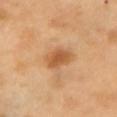<lesion>
  <biopsy_status>not biopsied; imaged during a skin examination</biopsy_status>
  <lesion_size>
    <long_diameter_mm_approx>3.5</long_diameter_mm_approx>
  </lesion_size>
  <image>
    <source>total-body photography crop</source>
    <field_of_view_mm>15</field_of_view_mm>
  </image>
  <site>chest</site>
  <patient>
    <sex>female</sex>
    <age_approx>55</age_approx>
  </patient>
</lesion>